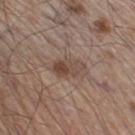Assessment:
The lesion was photographed on a routine skin check and not biopsied; there is no pathology result.
Acquisition and patient details:
This image is a 15 mm lesion crop taken from a total-body photograph. From the leg. The patient is a male approximately 60 years of age. Captured under white-light illumination.